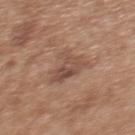Recorded during total-body skin imaging; not selected for excision or biopsy. A roughly 15 mm field-of-view crop from a total-body skin photograph. About 4.5 mm across. An algorithmic analysis of the crop reported about 8 CIELAB-L* units darker than the surrounding skin and a normalized lesion–skin contrast near 6.5. The analysis additionally found internal color variation of about 4.5 on a 0–10 scale and a peripheral color-asymmetry measure near 2. And it measured a classifier nevus-likeness of about 0/100. On the upper back. Imaged with white-light lighting. The patient is a female approximately 40 years of age.